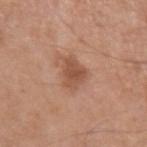Imaged during a routine full-body skin examination; the lesion was not biopsied and no histopathology is available. The lesion is on the arm. The subject is a male approximately 65 years of age. A 15 mm crop from a total-body photograph taken for skin-cancer surveillance.